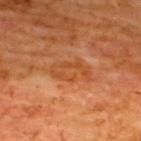Part of a total-body skin-imaging series; this lesion was reviewed on a skin check and was not flagged for biopsy. Imaged with cross-polarized lighting. The lesion's longest dimension is about 4.5 mm. A roughly 15 mm field-of-view crop from a total-body skin photograph. A male subject, in their mid- to late 60s. Located on the upper back.